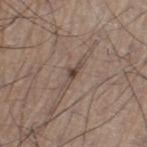No biopsy was performed on this lesion — it was imaged during a full skin examination and was not determined to be concerning. A 15 mm close-up extracted from a 3D total-body photography capture. The lesion is located on the left thigh. A male subject aged 58–62. This is a white-light tile.